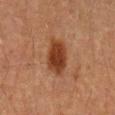Q: Was this lesion biopsied?
A: catalogued during a skin exam; not biopsied
Q: What lighting was used for the tile?
A: cross-polarized
Q: Where on the body is the lesion?
A: the mid back
Q: How large is the lesion?
A: ~5 mm (longest diameter)
Q: Patient demographics?
A: male, aged around 60
Q: What kind of image is this?
A: 15 mm crop, total-body photography